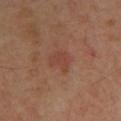| feature | finding |
|---|---|
| notes | no biopsy performed (imaged during a skin exam) |
| lighting | cross-polarized illumination |
| subject | male, approximately 55 years of age |
| site | the upper back |
| image-analysis metrics | an eccentricity of roughly 0.6 and two-axis asymmetry of about 0.35; a border-irregularity index near 3.5/10, a within-lesion color-variation index near 2.5/10, and a peripheral color-asymmetry measure near 1; an automated nevus-likeness rating near 5 out of 100 |
| image | 15 mm crop, total-body photography |
| lesion size | ≈3 mm |